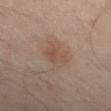| key | value |
|---|---|
| biopsy status | total-body-photography surveillance lesion; no biopsy |
| acquisition | ~15 mm crop, total-body skin-cancer survey |
| image-analysis metrics | a lesion–skin lightness drop of about 6 and a lesion-to-skin contrast of about 5 (normalized; higher = more distinct); a border-irregularity rating of about 3.5/10, a color-variation rating of about 3/10, and radial color variation of about 1 |
| location | the leg |
| subject | male, aged approximately 55 |
| lesion size | about 3 mm |